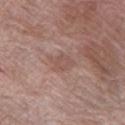Assessment: Imaged during a routine full-body skin examination; the lesion was not biopsied and no histopathology is available. Context: The subject is a female roughly 70 years of age. An algorithmic analysis of the crop reported a shape eccentricity near 0.55. The analysis additionally found a border-irregularity index near 2.5/10 and a within-lesion color-variation index near 1.5/10. From the leg. Cropped from a total-body skin-imaging series; the visible field is about 15 mm. Imaged with white-light lighting.